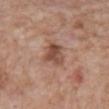{"biopsy_status": "not biopsied; imaged during a skin examination", "lighting": "white-light", "image": {"source": "total-body photography crop", "field_of_view_mm": 15}, "site": "abdomen", "patient": {"sex": "male", "age_approx": 60}, "lesion_size": {"long_diameter_mm_approx": 3.5}, "automated_metrics": {"area_mm2_approx": 7.5, "eccentricity": 0.6, "shape_asymmetry": 0.2, "cielab_L": 49, "cielab_a": 21, "cielab_b": 28, "vs_skin_darker_L": 11.0, "vs_skin_contrast_norm": 8.0, "lesion_detection_confidence_0_100": 100}}